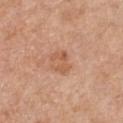biopsy status: catalogued during a skin exam; not biopsied
automated lesion analysis: a footprint of about 5 mm², an eccentricity of roughly 0.65, and a shape-asymmetry score of about 0.35 (0 = symmetric); a mean CIELAB color near L≈57 a*≈23 b*≈34, roughly 8 lightness units darker than nearby skin, and a normalized lesion–skin contrast near 6; an automated nevus-likeness rating near 5 out of 100
image: ~15 mm tile from a whole-body skin photo
site: the chest
patient: male, about 60 years old